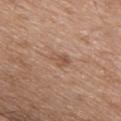Q: Was this lesion biopsied?
A: no biopsy performed (imaged during a skin exam)
Q: What lighting was used for the tile?
A: white-light
Q: Who is the patient?
A: male, aged approximately 50
Q: How was this image acquired?
A: 15 mm crop, total-body photography
Q: How large is the lesion?
A: about 3 mm
Q: Where on the body is the lesion?
A: the chest